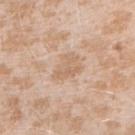workup: imaged on a skin check; not biopsied | acquisition: ~15 mm tile from a whole-body skin photo | automated lesion analysis: a footprint of about 7.5 mm² and a shape eccentricity near 0.75; an automated nevus-likeness rating near 0 out of 100 and a lesion-detection confidence of about 100/100 | patient: female, in their mid-20s | anatomic site: the right upper arm | size: ≈3.5 mm | tile lighting: white-light illumination.